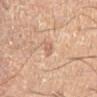| key | value |
|---|---|
| follow-up | catalogued during a skin exam; not biopsied |
| image | 15 mm crop, total-body photography |
| anatomic site | the left lower leg |
| subject | male, aged approximately 50 |
| diameter | about 2.5 mm |
| tile lighting | cross-polarized |